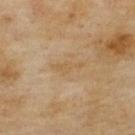Captured during whole-body skin photography for melanoma surveillance; the lesion was not biopsied. Measured at roughly 4 mm in maximum diameter. A 15 mm close-up tile from a total-body photography series done for melanoma screening. A female patient aged approximately 60. The tile uses cross-polarized illumination. The lesion is located on the upper back.